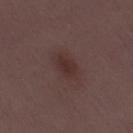notes: imaged on a skin check; not biopsied
site: the right thigh
imaging modality: total-body-photography crop, ~15 mm field of view
automated lesion analysis: a lesion area of about 4.5 mm², an eccentricity of roughly 0.8, and two-axis asymmetry of about 0.3; an average lesion color of about L≈30 a*≈18 b*≈18 (CIELAB) and a normalized border contrast of about 7
patient: female, aged around 30
lesion size: ~3 mm (longest diameter)